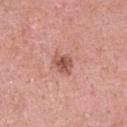notes: catalogued during a skin exam; not biopsied.